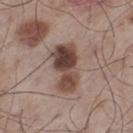Notes:
• follow-up · imaged on a skin check; not biopsied
• lesion size · ~6 mm (longest diameter)
• illumination · white-light illumination
• image · ~15 mm tile from a whole-body skin photo
• anatomic site · the left thigh
• subject · male, aged around 60